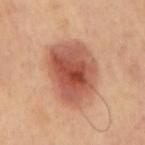Imaged during a routine full-body skin examination; the lesion was not biopsied and no histopathology is available.
This is a cross-polarized tile.
Measured at roughly 7.5 mm in maximum diameter.
On the mid back.
The lesion-visualizer software estimated an average lesion color of about L≈55 a*≈27 b*≈32 (CIELAB), a lesion–skin lightness drop of about 15, and a lesion-to-skin contrast of about 9.5 (normalized; higher = more distinct).
This image is a 15 mm lesion crop taken from a total-body photograph.